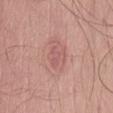Notes:
• workup · imaged on a skin check; not biopsied
• lesion size · ~2.5 mm (longest diameter)
• patient · male, roughly 60 years of age
• site · the abdomen
• illumination · white-light
• automated lesion analysis · a footprint of about 4.5 mm², a shape eccentricity near 0.4, and two-axis asymmetry of about 0.35; a lesion–skin lightness drop of about 7; a border-irregularity rating of about 3.5/10, internal color variation of about 2 on a 0–10 scale, and radial color variation of about 0.5
• image · ~15 mm tile from a whole-body skin photo